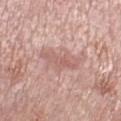{
  "biopsy_status": "not biopsied; imaged during a skin examination",
  "lighting": "white-light",
  "automated_metrics": {
    "area_mm2_approx": 7.5,
    "shape_asymmetry": 0.4,
    "vs_skin_darker_L": 8.0,
    "border_irregularity_0_10": 5.0,
    "color_variation_0_10": 2.5,
    "lesion_detection_confidence_0_100": 100
  },
  "lesion_size": {
    "long_diameter_mm_approx": 5.0
  },
  "image": {
    "source": "total-body photography crop",
    "field_of_view_mm": 15
  },
  "site": "right lower leg",
  "patient": {
    "sex": "female",
    "age_approx": 70
  }
}